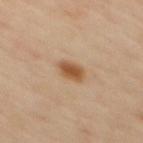Assessment:
The lesion was photographed on a routine skin check and not biopsied; there is no pathology result.
Background:
Located on the mid back. Automated image analysis of the tile measured a border-irregularity rating of about 2/10, internal color variation of about 2.5 on a 0–10 scale, and radial color variation of about 0.5. A 15 mm close-up tile from a total-body photography series done for melanoma screening. The patient is a male approximately 55 years of age.